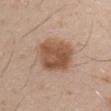Clinical impression: The lesion was photographed on a routine skin check and not biopsied; there is no pathology result. Image and clinical context: The lesion's longest dimension is about 5 mm. On the right upper arm. A 15 mm close-up tile from a total-body photography series done for melanoma screening. A male subject, aged 28 to 32. This is a white-light tile. An algorithmic analysis of the crop reported a lesion area of about 18 mm² and an outline eccentricity of about 0.55 (0 = round, 1 = elongated). The analysis additionally found roughly 12 lightness units darker than nearby skin. The software also gave a nevus-likeness score of about 85/100.